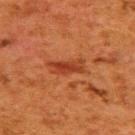Q: Was a biopsy performed?
A: imaged on a skin check; not biopsied
Q: What did automated image analysis measure?
A: a border-irregularity rating of about 3.5/10 and a within-lesion color-variation index near 4/10
Q: What are the patient's age and sex?
A: female, aged 48 to 52
Q: What lighting was used for the tile?
A: cross-polarized
Q: What is the anatomic site?
A: the upper back
Q: What is the lesion's diameter?
A: ~4.5 mm (longest diameter)
Q: How was this image acquired?
A: ~15 mm crop, total-body skin-cancer survey